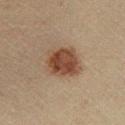<lesion>
  <lesion_size>
    <long_diameter_mm_approx>4.5</long_diameter_mm_approx>
  </lesion_size>
  <image>
    <source>total-body photography crop</source>
    <field_of_view_mm>15</field_of_view_mm>
  </image>
  <site>right lower leg</site>
  <patient>
    <sex>male</sex>
    <age_approx>45</age_approx>
  </patient>
  <automated_metrics>
    <border_irregularity_0_10>2.0</border_irregularity_0_10>
    <color_variation_0_10>4.0</color_variation_0_10>
    <peripheral_color_asymmetry>1.5</peripheral_color_asymmetry>
    <nevus_likeness_0_100>100</nevus_likeness_0_100>
    <lesion_detection_confidence_0_100>100</lesion_detection_confidence_0_100>
  </automated_metrics>
</lesion>